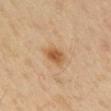Recorded during total-body skin imaging; not selected for excision or biopsy.
This image is a 15 mm lesion crop taken from a total-body photograph.
About 2.5 mm across.
The patient is a male approximately 40 years of age.
The lesion is located on the arm.
Captured under cross-polarized illumination.